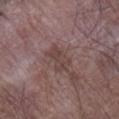Recorded during total-body skin imaging; not selected for excision or biopsy. An algorithmic analysis of the crop reported a mean CIELAB color near L≈42 a*≈17 b*≈19 and a normalized border contrast of about 6. Imaged with white-light lighting. Located on the right lower leg. Approximately 3.5 mm at its widest. A close-up tile cropped from a whole-body skin photograph, about 15 mm across. A male subject aged 78–82.